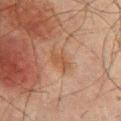Findings:
* workup — total-body-photography surveillance lesion; no biopsy
* subject — male, aged approximately 65
* image — ~15 mm tile from a whole-body skin photo
* size — ≈3 mm
* location — the upper back